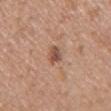Imaged with white-light lighting.
Located on the arm.
The recorded lesion diameter is about 2.5 mm.
A roughly 15 mm field-of-view crop from a total-body skin photograph.
A female patient in their 60s.